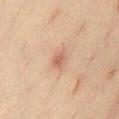Assessment: Recorded during total-body skin imaging; not selected for excision or biopsy. Background: From the abdomen. This image is a 15 mm lesion crop taken from a total-body photograph. A female subject, about 20 years old. Imaged with cross-polarized lighting. Longest diameter approximately 2.5 mm.